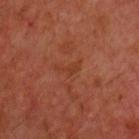Impression:
No biopsy was performed on this lesion — it was imaged during a full skin examination and was not determined to be concerning.
Image and clinical context:
This image is a 15 mm lesion crop taken from a total-body photograph. This is a cross-polarized tile. From the chest. A male subject, about 60 years old. The recorded lesion diameter is about 3 mm.